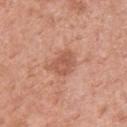Q: What are the patient's age and sex?
A: female, about 50 years old
Q: What kind of image is this?
A: 15 mm crop, total-body photography
Q: Where on the body is the lesion?
A: the upper back